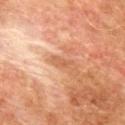{"biopsy_status": "not biopsied; imaged during a skin examination", "site": "mid back", "image": {"source": "total-body photography crop", "field_of_view_mm": 15}, "patient": {"sex": "male", "age_approx": 75}}